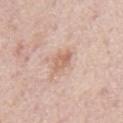Q: Was a biopsy performed?
A: catalogued during a skin exam; not biopsied
Q: What did automated image analysis measure?
A: an area of roughly 4.5 mm², a shape eccentricity near 0.75, and a shape-asymmetry score of about 0.3 (0 = symmetric); border irregularity of about 3.5 on a 0–10 scale and a peripheral color-asymmetry measure near 1.5; a nevus-likeness score of about 10/100 and a lesion-detection confidence of about 100/100
Q: Lesion location?
A: the mid back
Q: How was the tile lit?
A: white-light illumination
Q: What are the patient's age and sex?
A: male, in their mid-70s
Q: How large is the lesion?
A: ≈3 mm
Q: What is the imaging modality?
A: ~15 mm crop, total-body skin-cancer survey